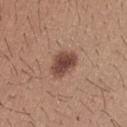{
  "biopsy_status": "not biopsied; imaged during a skin examination",
  "site": "upper back",
  "image": {
    "source": "total-body photography crop",
    "field_of_view_mm": 15
  },
  "patient": {
    "sex": "male",
    "age_approx": 25
  },
  "lighting": "white-light"
}